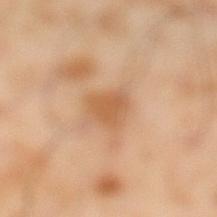Q: Is there a histopathology result?
A: no biopsy performed (imaged during a skin exam)